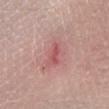Impression: No biopsy was performed on this lesion — it was imaged during a full skin examination and was not determined to be concerning. Acquisition and patient details: A close-up tile cropped from a whole-body skin photograph, about 15 mm across. The lesion is located on the left lower leg. The lesion's longest dimension is about 3 mm. Captured under white-light illumination. A female patient aged 43–47.